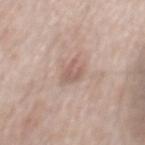| field | value |
|---|---|
| biopsy status | total-body-photography surveillance lesion; no biopsy |
| site | the mid back |
| TBP lesion metrics | a color-variation rating of about 1/10 and peripheral color asymmetry of about 0.5; a classifier nevus-likeness of about 5/100 and a lesion-detection confidence of about 100/100 |
| size | ~2.5 mm (longest diameter) |
| acquisition | 15 mm crop, total-body photography |
| patient | male, roughly 75 years of age |